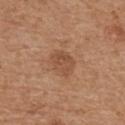No biopsy was performed on this lesion — it was imaged during a full skin examination and was not determined to be concerning.
Captured under white-light illumination.
An algorithmic analysis of the crop reported an area of roughly 6.5 mm² and an outline eccentricity of about 0.65 (0 = round, 1 = elongated). The analysis additionally found a lesion color around L≈50 a*≈22 b*≈32 in CIELAB, roughly 8 lightness units darker than nearby skin, and a lesion-to-skin contrast of about 6 (normalized; higher = more distinct). And it measured border irregularity of about 2.5 on a 0–10 scale, a within-lesion color-variation index near 3/10, and peripheral color asymmetry of about 1. The software also gave a classifier nevus-likeness of about 0/100 and lesion-presence confidence of about 100/100.
This image is a 15 mm lesion crop taken from a total-body photograph.
Measured at roughly 3 mm in maximum diameter.
A male subject aged 68 to 72.
The lesion is on the upper back.